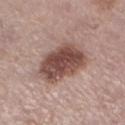workup = no biopsy performed (imaged during a skin exam) | lesion diameter = about 5.5 mm | tile lighting = white-light illumination | site = the leg | patient = female, roughly 65 years of age | acquisition = total-body-photography crop, ~15 mm field of view | automated metrics = a shape eccentricity near 0.6 and a symmetry-axis asymmetry near 0.2; a border-irregularity index near 2/10 and a peripheral color-asymmetry measure near 1.5.